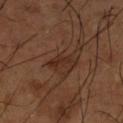<record>
  <lighting>cross-polarized</lighting>
  <site>left lower leg</site>
  <lesion_size>
    <long_diameter_mm_approx>4.0</long_diameter_mm_approx>
  </lesion_size>
  <image>
    <source>total-body photography crop</source>
    <field_of_view_mm>15</field_of_view_mm>
  </image>
  <patient>
    <sex>male</sex>
    <age_approx>65</age_approx>
  </patient>
  <automated_metrics>
    <area_mm2_approx>4.0</area_mm2_approx>
    <shape_asymmetry>0.4</shape_asymmetry>
    <cielab_L>28</cielab_L>
    <cielab_a>20</cielab_a>
    <cielab_b>25</cielab_b>
    <vs_skin_darker_L>7.0</vs_skin_darker_L>
    <vs_skin_contrast_norm>7.5</vs_skin_contrast_norm>
    <nevus_likeness_0_100>10</nevus_likeness_0_100>
    <lesion_detection_confidence_0_100>100</lesion_detection_confidence_0_100>
  </automated_metrics>
</record>